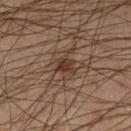{
  "automated_metrics": {
    "area_mm2_approx": 4.0
  },
  "patient": {
    "sex": "male",
    "age_approx": 45
  },
  "lighting": "cross-polarized",
  "lesion_size": {
    "long_diameter_mm_approx": 2.5
  },
  "image": {
    "source": "total-body photography crop",
    "field_of_view_mm": 15
  },
  "site": "left lower leg"
}